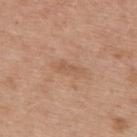Clinical impression: Recorded during total-body skin imaging; not selected for excision or biopsy. Acquisition and patient details: About 2.5 mm across. The tile uses white-light illumination. Cropped from a whole-body photographic skin survey; the tile spans about 15 mm. On the upper back. A female subject in their 40s.